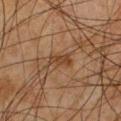{
  "biopsy_status": "not biopsied; imaged during a skin examination",
  "image": {
    "source": "total-body photography crop",
    "field_of_view_mm": 15
  },
  "site": "front of the torso",
  "patient": {
    "sex": "male",
    "age_approx": 50
  }
}